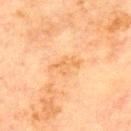A male subject, aged approximately 70.
This image is a 15 mm lesion crop taken from a total-body photograph.
On the chest.
Approximately 4 mm at its widest.
Captured under cross-polarized illumination.
An algorithmic analysis of the crop reported a footprint of about 5 mm² and a symmetry-axis asymmetry near 0.4. The analysis additionally found a border-irregularity index near 5.5/10, a color-variation rating of about 2/10, and radial color variation of about 1.